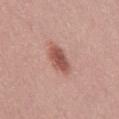Notes:
- biopsy status — total-body-photography surveillance lesion; no biopsy
- lesion size — about 4 mm
- anatomic site — the mid back
- patient — male, roughly 45 years of age
- tile lighting — white-light
- imaging modality — 15 mm crop, total-body photography